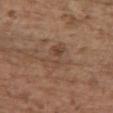<record>
  <biopsy_status>not biopsied; imaged during a skin examination</biopsy_status>
  <lighting>white-light</lighting>
  <lesion_size>
    <long_diameter_mm_approx>4.5</long_diameter_mm_approx>
  </lesion_size>
  <image>
    <source>total-body photography crop</source>
    <field_of_view_mm>15</field_of_view_mm>
  </image>
  <patient>
    <sex>female</sex>
    <age_approx>65</age_approx>
  </patient>
  <site>upper back</site>
</record>